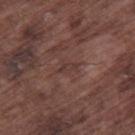Notes:
– follow-up: no biopsy performed (imaged during a skin exam)
– site: the leg
– TBP lesion metrics: a footprint of about 3.5 mm², a shape eccentricity near 0.75, and a symmetry-axis asymmetry near 0.45; an average lesion color of about L≈37 a*≈18 b*≈21 (CIELAB), a lesion–skin lightness drop of about 5, and a normalized lesion–skin contrast near 4.5; a border-irregularity rating of about 5/10, internal color variation of about 1.5 on a 0–10 scale, and a peripheral color-asymmetry measure near 0.5
– lesion diameter: about 2.5 mm
– patient: male, aged 73 to 77
– acquisition: 15 mm crop, total-body photography